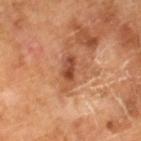Notes:
• biopsy status — catalogued during a skin exam; not biopsied
• lighting — cross-polarized illumination
• subject — male, aged 63 to 67
• lesion size — ≈4 mm
• image — ~15 mm crop, total-body skin-cancer survey